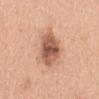Q: Was this lesion biopsied?
A: imaged on a skin check; not biopsied
Q: Who is the patient?
A: female, aged approximately 55
Q: Where on the body is the lesion?
A: the abdomen
Q: What kind of image is this?
A: ~15 mm crop, total-body skin-cancer survey
Q: How large is the lesion?
A: ≈5 mm
Q: What lighting was used for the tile?
A: white-light illumination